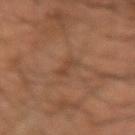The subject is a male about 50 years old.
Imaged with cross-polarized lighting.
A close-up tile cropped from a whole-body skin photograph, about 15 mm across.
Located on the right forearm.
Longest diameter approximately 3 mm.